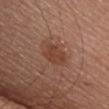Impression: Part of a total-body skin-imaging series; this lesion was reviewed on a skin check and was not flagged for biopsy. Context: Imaged with white-light lighting. From the chest. A lesion tile, about 15 mm wide, cut from a 3D total-body photograph. About 4 mm across. A female subject, in their mid- to late 50s. The total-body-photography lesion software estimated a border-irregularity index near 2.5/10, a within-lesion color-variation index near 3/10, and a peripheral color-asymmetry measure near 1. The analysis additionally found a detector confidence of about 100 out of 100 that the crop contains a lesion.